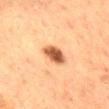No biopsy was performed on this lesion — it was imaged during a full skin examination and was not determined to be concerning.
From the mid back.
The total-body-photography lesion software estimated border irregularity of about 1.5 on a 0–10 scale, a color-variation rating of about 4/10, and peripheral color asymmetry of about 1. The software also gave a lesion-detection confidence of about 100/100.
The lesion's longest dimension is about 3 mm.
A 15 mm close-up extracted from a 3D total-body photography capture.
The subject is a female roughly 55 years of age.